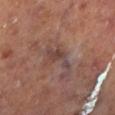Assessment: The lesion was tiled from a total-body skin photograph and was not biopsied.